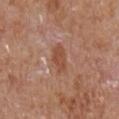  biopsy_status: not biopsied; imaged during a skin examination
  site: chest
  patient:
    sex: male
    age_approx: 65
  lighting: white-light
  image:
    source: total-body photography crop
    field_of_view_mm: 15
  lesion_size:
    long_diameter_mm_approx: 3.5